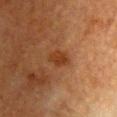Case summary:
* notes · imaged on a skin check; not biopsied
* image · 15 mm crop, total-body photography
* body site · the chest
* subject · female, aged around 55
* image-analysis metrics · a lesion area of about 4.5 mm², an eccentricity of roughly 0.7, and a symmetry-axis asymmetry near 0.15; about 7 CIELAB-L* units darker than the surrounding skin; internal color variation of about 2 on a 0–10 scale and a peripheral color-asymmetry measure near 0.5
* illumination · cross-polarized